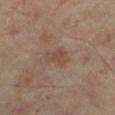{"lesion_size": {"long_diameter_mm_approx": 2.5}, "automated_metrics": {"area_mm2_approx": 4.5, "eccentricity": 0.7, "shape_asymmetry": 0.4, "cielab_L": 44, "cielab_a": 16, "cielab_b": 25, "vs_skin_darker_L": 6.0, "vs_skin_contrast_norm": 5.5}, "lighting": "cross-polarized", "image": {"source": "total-body photography crop", "field_of_view_mm": 15}, "site": "right lower leg", "patient": {"sex": "male", "age_approx": 70}}